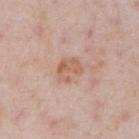workup = catalogued during a skin exam; not biopsied | anatomic site = the chest | size = ≈3 mm | lighting = white-light illumination | image source = total-body-photography crop, ~15 mm field of view | patient = male, in their mid-70s | automated lesion analysis = a lesion color around L≈60 a*≈20 b*≈29 in CIELAB; border irregularity of about 4.5 on a 0–10 scale, internal color variation of about 3.5 on a 0–10 scale, and peripheral color asymmetry of about 1.